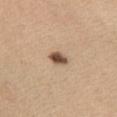The lesion was tiled from a total-body skin photograph and was not biopsied. This is a white-light tile. The lesion is located on the left thigh. A 15 mm close-up extracted from a 3D total-body photography capture. The lesion's longest dimension is about 2.5 mm. The lesion-visualizer software estimated a color-variation rating of about 4/10 and peripheral color asymmetry of about 1.5. The analysis additionally found an automated nevus-likeness rating near 100 out of 100 and lesion-presence confidence of about 100/100. A female patient, aged around 60.